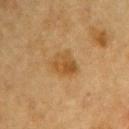- notes — total-body-photography surveillance lesion; no biopsy
- diameter — about 3.5 mm
- image — total-body-photography crop, ~15 mm field of view
- subject — male, approximately 85 years of age or older
- location — the chest